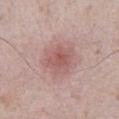Impression:
Imaged during a routine full-body skin examination; the lesion was not biopsied and no histopathology is available.
Acquisition and patient details:
The patient is a male aged approximately 80. Located on the front of the torso. Cropped from a whole-body photographic skin survey; the tile spans about 15 mm.